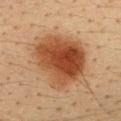Imaged during a routine full-body skin examination; the lesion was not biopsied and no histopathology is available. A roughly 15 mm field-of-view crop from a total-body skin photograph. On the upper back. The patient is a male roughly 35 years of age. The tile uses cross-polarized illumination.